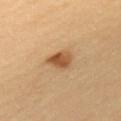Case summary:
– biopsy status: imaged on a skin check; not biopsied
– imaging modality: ~15 mm tile from a whole-body skin photo
– site: the left upper arm
– subject: male, aged around 40
– illumination: cross-polarized
– lesion diameter: about 3 mm